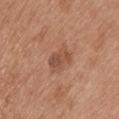The lesion was tiled from a total-body skin photograph and was not biopsied. On the upper back. A 15 mm close-up tile from a total-body photography series done for melanoma screening. Captured under white-light illumination. A male subject in their mid-60s. Measured at roughly 3.5 mm in maximum diameter.